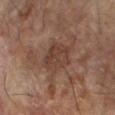follow-up: imaged on a skin check; not biopsied | imaging modality: 15 mm crop, total-body photography | location: the right forearm | patient: male, approximately 65 years of age.